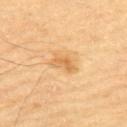This lesion was catalogued during total-body skin photography and was not selected for biopsy. Imaged with cross-polarized lighting. The lesion-visualizer software estimated a border-irregularity rating of about 4/10, a within-lesion color-variation index near 2.5/10, and radial color variation of about 1. The software also gave a classifier nevus-likeness of about 15/100 and a lesion-detection confidence of about 100/100. The lesion is located on the upper back. A close-up tile cropped from a whole-body skin photograph, about 15 mm across. A male subject in their mid- to late 80s or older.